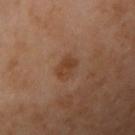Impression:
Part of a total-body skin-imaging series; this lesion was reviewed on a skin check and was not flagged for biopsy.
Image and clinical context:
A lesion tile, about 15 mm wide, cut from a 3D total-body photograph. Located on the right upper arm. A female subject roughly 55 years of age. Measured at roughly 2.5 mm in maximum diameter. Imaged with cross-polarized lighting.